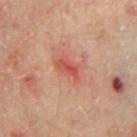No biopsy was performed on this lesion — it was imaged during a full skin examination and was not determined to be concerning. Located on the mid back. A 15 mm crop from a total-body photograph taken for skin-cancer surveillance. The lesion's longest dimension is about 3 mm. Imaged with cross-polarized lighting. The total-body-photography lesion software estimated a lesion area of about 3.5 mm² and a shape-asymmetry score of about 0.35 (0 = symmetric). And it measured a mean CIELAB color near L≈52 a*≈34 b*≈31 and a lesion–skin lightness drop of about 9. The software also gave a border-irregularity rating of about 3.5/10, a color-variation rating of about 2.5/10, and radial color variation of about 0.5. A male subject, about 65 years old.